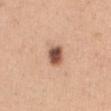notes: no biopsy performed (imaged during a skin exam) | anatomic site: the front of the torso | lighting: white-light illumination | image source: 15 mm crop, total-body photography | subject: female, in their 30s.